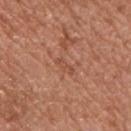Case summary:
– workup: catalogued during a skin exam; not biopsied
– patient: male, approximately 55 years of age
– image-analysis metrics: a lesion area of about 2 mm², an outline eccentricity of about 0.95 (0 = round, 1 = elongated), and a symmetry-axis asymmetry near 0.4
– illumination: white-light illumination
– imaging modality: ~15 mm crop, total-body skin-cancer survey
– body site: the chest
– size: about 2.5 mm